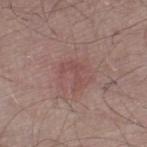<case>
<biopsy_status>not biopsied; imaged during a skin examination</biopsy_status>
<site>left thigh</site>
<patient>
  <sex>male</sex>
  <age_approx>55</age_approx>
</patient>
<lighting>white-light</lighting>
<automated_metrics>
  <area_mm2_approx>4.5</area_mm2_approx>
  <eccentricity>0.8</eccentricity>
  <nevus_likeness_0_100>0</nevus_likeness_0_100>
  <lesion_detection_confidence_0_100>100</lesion_detection_confidence_0_100>
</automated_metrics>
<image>
  <source>total-body photography crop</source>
  <field_of_view_mm>15</field_of_view_mm>
</image>
<lesion_size>
  <long_diameter_mm_approx>3.5</long_diameter_mm_approx>
</lesion_size>
</case>